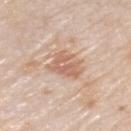The lesion was photographed on a routine skin check and not biopsied; there is no pathology result.
From the left upper arm.
A region of skin cropped from a whole-body photographic capture, roughly 15 mm wide.
The tile uses white-light illumination.
Longest diameter approximately 3.5 mm.
A male patient in their mid- to late 70s.